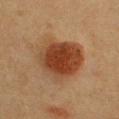Image and clinical context: Imaged with cross-polarized lighting. A 15 mm close-up extracted from a 3D total-body photography capture. The patient is a female roughly 40 years of age. From the chest.